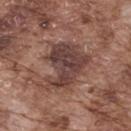Image and clinical context:
Measured at roughly 6.5 mm in maximum diameter. This is a white-light tile. The lesion is located on the upper back. A 15 mm close-up extracted from a 3D total-body photography capture. A male subject in their mid- to late 70s.